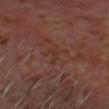Captured during whole-body skin photography for melanoma surveillance; the lesion was not biopsied.